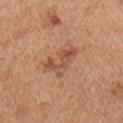No biopsy was performed on this lesion — it was imaged during a full skin examination and was not determined to be concerning.
Automated image analysis of the tile measured a footprint of about 7 mm², an outline eccentricity of about 0.85 (0 = round, 1 = elongated), and two-axis asymmetry of about 0.5. The analysis additionally found a border-irregularity rating of about 8/10 and internal color variation of about 2.5 on a 0–10 scale. And it measured lesion-presence confidence of about 100/100.
A male subject, in their mid- to late 60s.
A close-up tile cropped from a whole-body skin photograph, about 15 mm across.
Approximately 4.5 mm at its widest.
Captured under white-light illumination.
On the right upper arm.